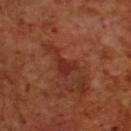Captured during whole-body skin photography for melanoma surveillance; the lesion was not biopsied.
A lesion tile, about 15 mm wide, cut from a 3D total-body photograph.
The recorded lesion diameter is about 4 mm.
The lesion is located on the upper back.
The patient is a male aged 68 to 72.
Imaged with cross-polarized lighting.